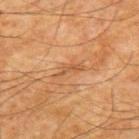No biopsy was performed on this lesion — it was imaged during a full skin examination and was not determined to be concerning. A roughly 15 mm field-of-view crop from a total-body skin photograph. Imaged with cross-polarized lighting. From the upper back. Automated tile analysis of the lesion measured a within-lesion color-variation index near 0/10 and peripheral color asymmetry of about 0. A male patient aged 63 to 67.